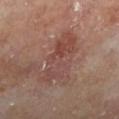  biopsy_status: not biopsied; imaged during a skin examination
  site: leg
  patient:
    sex: male
    age_approx: 65
  image:
    source: total-body photography crop
    field_of_view_mm: 15
  automated_metrics:
    cielab_L: 43
    cielab_a: 20
    cielab_b: 23
    vs_skin_contrast_norm: 6.0
  lighting: cross-polarized